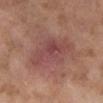The lesion was photographed on a routine skin check and not biopsied; there is no pathology result. A 15 mm close-up tile from a total-body photography series done for melanoma screening. From the right lower leg. Captured under cross-polarized illumination. A female subject, aged 58–62. Automated image analysis of the tile measured border irregularity of about 4 on a 0–10 scale and peripheral color asymmetry of about 1. It also reported a classifier nevus-likeness of about 0/100 and a detector confidence of about 100 out of 100 that the crop contains a lesion.